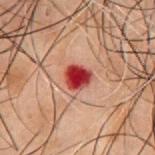lesion diameter: ≈3 mm
lighting: cross-polarized
imaging modality: 15 mm crop, total-body photography
patient: male, about 50 years old
location: the chest
automated lesion analysis: a shape eccentricity near 0.5 and a shape-asymmetry score of about 0.2 (0 = symmetric); about 16 CIELAB-L* units darker than the surrounding skin and a lesion-to-skin contrast of about 14 (normalized; higher = more distinct); peripheral color asymmetry of about 1.5; a nevus-likeness score of about 0/100 and lesion-presence confidence of about 100/100